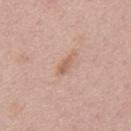This lesion was catalogued during total-body skin photography and was not selected for biopsy. A male subject, approximately 45 years of age. From the mid back. A lesion tile, about 15 mm wide, cut from a 3D total-body photograph.